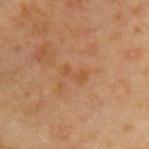The lesion was photographed on a routine skin check and not biopsied; there is no pathology result. Captured under cross-polarized illumination. The recorded lesion diameter is about 3 mm. On the chest. A male subject, aged approximately 65. A region of skin cropped from a whole-body photographic capture, roughly 15 mm wide. The total-body-photography lesion software estimated two-axis asymmetry of about 0.35. The software also gave a border-irregularity index near 4.5/10, a within-lesion color-variation index near 0/10, and a peripheral color-asymmetry measure near 0. The software also gave a nevus-likeness score of about 0/100 and a detector confidence of about 100 out of 100 that the crop contains a lesion.